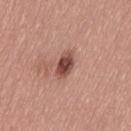Recorded during total-body skin imaging; not selected for excision or biopsy.
This image is a 15 mm lesion crop taken from a total-body photograph.
Automated tile analysis of the lesion measured a lesion color around L≈47 a*≈23 b*≈26 in CIELAB, roughly 16 lightness units darker than nearby skin, and a lesion-to-skin contrast of about 11 (normalized; higher = more distinct). It also reported border irregularity of about 1.5 on a 0–10 scale and radial color variation of about 1.5. The analysis additionally found a classifier nevus-likeness of about 90/100.
From the left thigh.
The subject is a male roughly 55 years of age.
This is a white-light tile.
Measured at roughly 3.5 mm in maximum diameter.